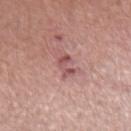Captured during whole-body skin photography for melanoma surveillance; the lesion was not biopsied.
Captured under white-light illumination.
A roughly 15 mm field-of-view crop from a total-body skin photograph.
A male subject aged around 55.
On the right forearm.
Approximately 3 mm at its widest.